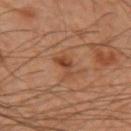Part of a total-body skin-imaging series; this lesion was reviewed on a skin check and was not flagged for biopsy. The lesion's longest dimension is about 3.5 mm. Imaged with cross-polarized lighting. A male subject, aged around 50. A lesion tile, about 15 mm wide, cut from a 3D total-body photograph. The lesion is on the left forearm.